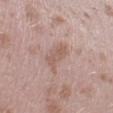| feature | finding |
|---|---|
| biopsy status | no biopsy performed (imaged during a skin exam) |
| lighting | white-light |
| acquisition | ~15 mm tile from a whole-body skin photo |
| body site | the right lower leg |
| patient | female, roughly 25 years of age |
| lesion size | about 4.5 mm |